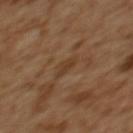Q: Was a biopsy performed?
A: total-body-photography surveillance lesion; no biopsy
Q: What is the imaging modality?
A: 15 mm crop, total-body photography
Q: What is the anatomic site?
A: the upper back
Q: How large is the lesion?
A: ≈2.5 mm
Q: What did automated image analysis measure?
A: a footprint of about 3 mm², a shape eccentricity near 0.8, and two-axis asymmetry of about 0.4; a mean CIELAB color near L≈39 a*≈17 b*≈32, a lesion–skin lightness drop of about 6, and a lesion-to-skin contrast of about 6 (normalized; higher = more distinct); border irregularity of about 4 on a 0–10 scale, a color-variation rating of about 1/10, and peripheral color asymmetry of about 0; a classifier nevus-likeness of about 0/100 and lesion-presence confidence of about 85/100
Q: Patient demographics?
A: female, about 55 years old
Q: Illumination type?
A: cross-polarized illumination